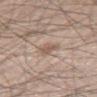<tbp_lesion>
<biopsy_status>not biopsied; imaged during a skin examination</biopsy_status>
<lesion_size>
  <long_diameter_mm_approx>2.5</long_diameter_mm_approx>
</lesion_size>
<image>
  <source>total-body photography crop</source>
  <field_of_view_mm>15</field_of_view_mm>
</image>
<patient>
  <sex>male</sex>
  <age_approx>45</age_approx>
</patient>
<site>right thigh</site>
<automated_metrics>
  <area_mm2_approx>4.0</area_mm2_approx>
  <eccentricity>0.65</eccentricity>
  <shape_asymmetry>0.25</shape_asymmetry>
  <cielab_L>57</cielab_L>
  <cielab_a>16</cielab_a>
  <cielab_b>26</cielab_b>
  <vs_skin_darker_L>9.0</vs_skin_darker_L>
  <vs_skin_contrast_norm>6.0</vs_skin_contrast_norm>
  <nevus_likeness_0_100>35</nevus_likeness_0_100>
  <lesion_detection_confidence_0_100>100</lesion_detection_confidence_0_100>
</automated_metrics>
</tbp_lesion>